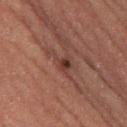No biopsy was performed on this lesion — it was imaged during a full skin examination and was not determined to be concerning.
The lesion's longest dimension is about 4 mm.
A male patient, in their 60s.
The lesion is located on the left thigh.
A 15 mm close-up extracted from a 3D total-body photography capture.
Imaged with cross-polarized lighting.